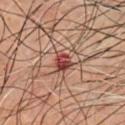workup — imaged on a skin check; not biopsied
patient — male, approximately 65 years of age
body site — the chest
image source — total-body-photography crop, ~15 mm field of view